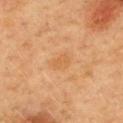Q: Was a biopsy performed?
A: imaged on a skin check; not biopsied
Q: What kind of image is this?
A: total-body-photography crop, ~15 mm field of view
Q: What lighting was used for the tile?
A: cross-polarized illumination
Q: Who is the patient?
A: female, aged 58–62
Q: Lesion location?
A: the upper back
Q: Lesion size?
A: about 3 mm